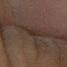| field | value |
|---|---|
| notes | imaged on a skin check; not biopsied |
| anatomic site | the left forearm |
| lighting | cross-polarized illumination |
| patient | male, aged 48 to 52 |
| imaging modality | ~15 mm crop, total-body skin-cancer survey |
| lesion diameter | about 3 mm |
| image-analysis metrics | a footprint of about 2.5 mm², a shape eccentricity near 0.95, and a symmetry-axis asymmetry near 0.3; a lesion color around L≈21 a*≈10 b*≈15 in CIELAB, roughly 4 lightness units darker than nearby skin, and a normalized lesion–skin contrast near 5.5; a border-irregularity rating of about 3/10, a color-variation rating of about 0/10, and radial color variation of about 0 |